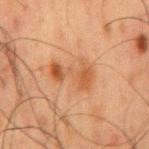Q: Was this lesion biopsied?
A: no biopsy performed (imaged during a skin exam)
Q: What are the patient's age and sex?
A: male, about 60 years old
Q: Automated lesion metrics?
A: a lesion color around L≈44 a*≈21 b*≈32 in CIELAB and a lesion–skin lightness drop of about 7; a border-irregularity index near 7/10, internal color variation of about 3.5 on a 0–10 scale, and radial color variation of about 1; an automated nevus-likeness rating near 80 out of 100 and lesion-presence confidence of about 100/100
Q: Lesion location?
A: the back
Q: How large is the lesion?
A: about 5 mm
Q: How was this image acquired?
A: 15 mm crop, total-body photography
Q: What lighting was used for the tile?
A: cross-polarized illumination